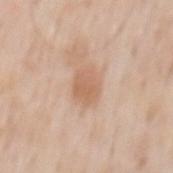  biopsy_status: not biopsied; imaged during a skin examination